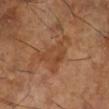Impression: The lesion was photographed on a routine skin check and not biopsied; there is no pathology result. Background: About 5 mm across. The patient is a male about 65 years old. A roughly 15 mm field-of-view crop from a total-body skin photograph. Located on the right lower leg. This is a cross-polarized tile.